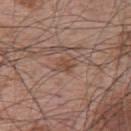notes: catalogued during a skin exam; not biopsied | lesion size: ~2.5 mm (longest diameter) | image: ~15 mm crop, total-body skin-cancer survey | location: the upper back | TBP lesion metrics: a lesion color around L≈48 a*≈19 b*≈27 in CIELAB, a lesion–skin lightness drop of about 7, and a lesion-to-skin contrast of about 6 (normalized; higher = more distinct); a nevus-likeness score of about 0/100 and a detector confidence of about 95 out of 100 that the crop contains a lesion | tile lighting: white-light illumination | patient: male, roughly 65 years of age.